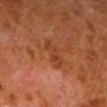workup = total-body-photography surveillance lesion; no biopsy
tile lighting = cross-polarized
image source = ~15 mm crop, total-body skin-cancer survey
subject = male, aged approximately 80
body site = the right lower leg